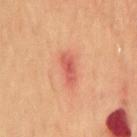Imaged during a routine full-body skin examination; the lesion was not biopsied and no histopathology is available.